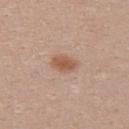This lesion was catalogued during total-body skin photography and was not selected for biopsy. On the upper back. The patient is a female approximately 25 years of age. Cropped from a whole-body photographic skin survey; the tile spans about 15 mm. Captured under white-light illumination.